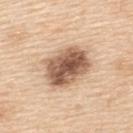Recorded during total-body skin imaging; not selected for excision or biopsy. A female patient, about 55 years old. On the upper back. A region of skin cropped from a whole-body photographic capture, roughly 15 mm wide.